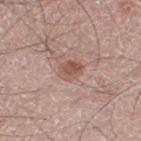The lesion was photographed on a routine skin check and not biopsied; there is no pathology result.
A male subject aged 48 to 52.
A 15 mm close-up extracted from a 3D total-body photography capture.
The lesion's longest dimension is about 2.5 mm.
Captured under white-light illumination.
The lesion is on the left thigh.